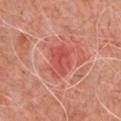<record>
<biopsy_status>not biopsied; imaged during a skin examination</biopsy_status>
<lighting>cross-polarized</lighting>
<site>chest</site>
<image>
  <source>total-body photography crop</source>
  <field_of_view_mm>15</field_of_view_mm>
</image>
<patient>
  <sex>male</sex>
  <age_approx>50</age_approx>
</patient>
</record>